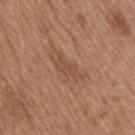follow-up: total-body-photography surveillance lesion; no biopsy | size: ~4 mm (longest diameter) | location: the upper back | automated lesion analysis: an area of roughly 5.5 mm² and a symmetry-axis asymmetry near 0.45; a lesion color around L≈49 a*≈21 b*≈30 in CIELAB, roughly 7 lightness units darker than nearby skin, and a normalized lesion–skin contrast near 5; internal color variation of about 2 on a 0–10 scale and a peripheral color-asymmetry measure near 1; a nevus-likeness score of about 15/100 and a lesion-detection confidence of about 100/100 | image: ~15 mm crop, total-body skin-cancer survey | subject: male, aged approximately 65.